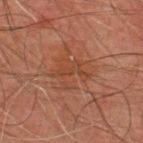Part of a total-body skin-imaging series; this lesion was reviewed on a skin check and was not flagged for biopsy. A 15 mm close-up extracted from a 3D total-body photography capture. Longest diameter approximately 4 mm. The tile uses cross-polarized illumination. The subject is a male approximately 45 years of age. The lesion is on the upper back.